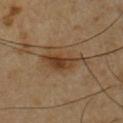The total-body-photography lesion software estimated a shape eccentricity near 0.9 and a symmetry-axis asymmetry near 0.45. The analysis additionally found about 9 CIELAB-L* units darker than the surrounding skin and a normalized border contrast of about 8. It also reported a border-irregularity rating of about 6.5/10 and radial color variation of about 1.5. The software also gave a classifier nevus-likeness of about 85/100 and lesion-presence confidence of about 100/100. On the chest. Captured under cross-polarized illumination. The subject is a male aged approximately 55. A 15 mm crop from a total-body photograph taken for skin-cancer surveillance.